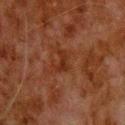Impression:
Recorded during total-body skin imaging; not selected for excision or biopsy.
Background:
A male patient, aged 78–82. A roughly 15 mm field-of-view crop from a total-body skin photograph. On the upper back.